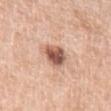biopsy status — catalogued during a skin exam; not biopsied | tile lighting — white-light illumination | subject — male, aged approximately 60 | acquisition — total-body-photography crop, ~15 mm field of view | automated metrics — an area of roughly 6.5 mm² and an outline eccentricity of about 0.65 (0 = round, 1 = elongated) | location — the left upper arm.